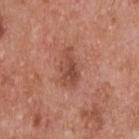Clinical summary:
A lesion tile, about 15 mm wide, cut from a 3D total-body photograph. Located on the upper back. The tile uses white-light illumination. The total-body-photography lesion software estimated an outline eccentricity of about 0.85 (0 = round, 1 = elongated). A male subject roughly 55 years of age.